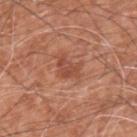{"biopsy_status": "not biopsied; imaged during a skin examination", "automated_metrics": {"area_mm2_approx": 5.5, "eccentricity": 0.7, "shape_asymmetry": 0.4, "cielab_L": 49, "cielab_a": 26, "cielab_b": 32, "vs_skin_darker_L": 8.0, "vs_skin_contrast_norm": 6.0, "nevus_likeness_0_100": 5, "lesion_detection_confidence_0_100": 100}, "lighting": "white-light", "patient": {"sex": "male", "age_approx": 60}, "site": "back", "lesion_size": {"long_diameter_mm_approx": 3.0}, "image": {"source": "total-body photography crop", "field_of_view_mm": 15}}